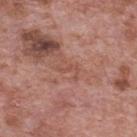Impression:
This lesion was catalogued during total-body skin photography and was not selected for biopsy.
Context:
A male patient aged around 70. A lesion tile, about 15 mm wide, cut from a 3D total-body photograph. The recorded lesion diameter is about 2.5 mm. Located on the mid back. The tile uses white-light illumination.